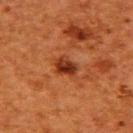Q: Is there a histopathology result?
A: total-body-photography surveillance lesion; no biopsy
Q: What kind of image is this?
A: total-body-photography crop, ~15 mm field of view
Q: How large is the lesion?
A: ~3 mm (longest diameter)
Q: Patient demographics?
A: female, approximately 50 years of age
Q: What is the anatomic site?
A: the upper back